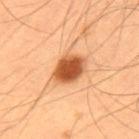| feature | finding |
|---|---|
| location | the upper back |
| imaging modality | 15 mm crop, total-body photography |
| patient | male, aged approximately 55 |
| automated metrics | a mean CIELAB color near L≈55 a*≈28 b*≈41, about 18 CIELAB-L* units darker than the surrounding skin, and a normalized border contrast of about 11.5 |